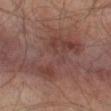<record>
<biopsy_status>not biopsied; imaged during a skin examination</biopsy_status>
<site>left thigh</site>
<lesion_size>
  <long_diameter_mm_approx>8.5</long_diameter_mm_approx>
</lesion_size>
<image>
  <source>total-body photography crop</source>
  <field_of_view_mm>15</field_of_view_mm>
</image>
<patient>
  <sex>male</sex>
  <age_approx>65</age_approx>
</patient>
<lighting>cross-polarized</lighting>
<automated_metrics>
  <area_mm2_approx>24.0</area_mm2_approx>
  <eccentricity>0.85</eccentricity>
  <shape_asymmetry>0.6</shape_asymmetry>
  <cielab_L>34</cielab_L>
  <cielab_a>17</cielab_a>
  <cielab_b>19</cielab_b>
  <border_irregularity_0_10>9.5</border_irregularity_0_10>
  <nevus_likeness_0_100>0</nevus_likeness_0_100>
  <lesion_detection_confidence_0_100>90</lesion_detection_confidence_0_100>
</automated_metrics>
</record>